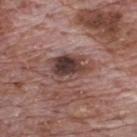This lesion was catalogued during total-body skin photography and was not selected for biopsy. The total-body-photography lesion software estimated a lesion color around L≈40 a*≈19 b*≈20 in CIELAB, a lesion–skin lightness drop of about 13, and a normalized border contrast of about 10.5. The analysis additionally found a border-irregularity rating of about 3.5/10, a within-lesion color-variation index near 9.5/10, and peripheral color asymmetry of about 3.5. The software also gave a classifier nevus-likeness of about 0/100 and lesion-presence confidence of about 100/100. The subject is a male approximately 70 years of age. This is a white-light tile. The recorded lesion diameter is about 5 mm. The lesion is on the back. A 15 mm crop from a total-body photograph taken for skin-cancer surveillance.